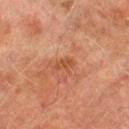Part of a total-body skin-imaging series; this lesion was reviewed on a skin check and was not flagged for biopsy. An algorithmic analysis of the crop reported a lesion area of about 3 mm², an outline eccentricity of about 0.9 (0 = round, 1 = elongated), and a symmetry-axis asymmetry near 0.4. And it measured roughly 7 lightness units darker than nearby skin. A roughly 15 mm field-of-view crop from a total-body skin photograph. A male patient in their mid-70s. From the right lower leg.